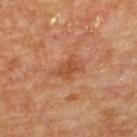{
  "biopsy_status": "not biopsied; imaged during a skin examination",
  "patient": {
    "sex": "male",
    "age_approx": "85+"
  },
  "automated_metrics": {
    "border_irregularity_0_10": 4.5,
    "color_variation_0_10": 2.0,
    "peripheral_color_asymmetry": 1.0,
    "nevus_likeness_0_100": 0
  },
  "lesion_size": {
    "long_diameter_mm_approx": 3.5
  },
  "image": {
    "source": "total-body photography crop",
    "field_of_view_mm": 15
  },
  "site": "upper back"
}